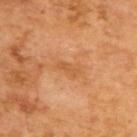biopsy_status: not biopsied; imaged during a skin examination
site: upper back
image:
  source: total-body photography crop
  field_of_view_mm: 15
lesion_size:
  long_diameter_mm_approx: 2.5
patient:
  sex: male
  age_approx: 65